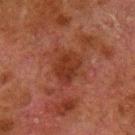Context: A male patient aged 78–82. The total-body-photography lesion software estimated an eccentricity of roughly 0.65 and a symmetry-axis asymmetry near 0.25. The analysis additionally found border irregularity of about 2.5 on a 0–10 scale, internal color variation of about 2.5 on a 0–10 scale, and radial color variation of about 1. Located on the left lower leg. A 15 mm crop from a total-body photograph taken for skin-cancer surveillance. This is a cross-polarized tile.